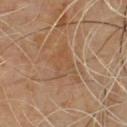The tile uses cross-polarized illumination. From the chest. A male patient in their mid- to late 40s. This image is a 15 mm lesion crop taken from a total-body photograph. The lesion-visualizer software estimated a lesion area of about 10 mm² and a symmetry-axis asymmetry near 0.45. And it measured a normalized border contrast of about 4.5. Approximately 5 mm at its widest.